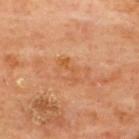The patient is a male aged approximately 65. Imaged with cross-polarized lighting. On the upper back. Cropped from a whole-body photographic skin survey; the tile spans about 15 mm. Approximately 3 mm at its widest.